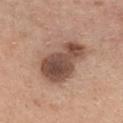Assessment: No biopsy was performed on this lesion — it was imaged during a full skin examination and was not determined to be concerning.